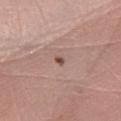Q: Was a biopsy performed?
A: catalogued during a skin exam; not biopsied